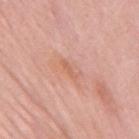{"biopsy_status": "not biopsied; imaged during a skin examination"}